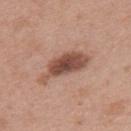Impression:
Part of a total-body skin-imaging series; this lesion was reviewed on a skin check and was not flagged for biopsy.
Background:
The lesion's longest dimension is about 6 mm. A female subject, aged around 40. Automated tile analysis of the lesion measured an area of roughly 12 mm², an outline eccentricity of about 0.9 (0 = round, 1 = elongated), and two-axis asymmetry of about 0.35. And it measured a border-irregularity rating of about 4.5/10, a within-lesion color-variation index near 4/10, and peripheral color asymmetry of about 1. The software also gave an automated nevus-likeness rating near 90 out of 100. On the upper back. A lesion tile, about 15 mm wide, cut from a 3D total-body photograph.